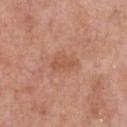No biopsy was performed on this lesion — it was imaged during a full skin examination and was not determined to be concerning. A lesion tile, about 15 mm wide, cut from a 3D total-body photograph. Located on the chest. A male patient, roughly 80 years of age. Captured under white-light illumination. Approximately 3.5 mm at its widest.